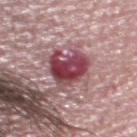Q: Is there a histopathology result?
A: catalogued during a skin exam; not biopsied
Q: Patient demographics?
A: male, roughly 35 years of age
Q: What is the imaging modality?
A: total-body-photography crop, ~15 mm field of view
Q: How was the tile lit?
A: white-light
Q: Where on the body is the lesion?
A: the head or neck
Q: What is the lesion's diameter?
A: ~5.5 mm (longest diameter)